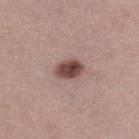Imaged during a routine full-body skin examination; the lesion was not biopsied and no histopathology is available. On the right lower leg. The subject is a female aged approximately 50. Captured under white-light illumination. A roughly 15 mm field-of-view crop from a total-body skin photograph.